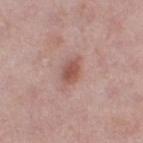notes = imaged on a skin check; not biopsied | subject = female, roughly 50 years of age | acquisition = 15 mm crop, total-body photography | anatomic site = the right thigh | image-analysis metrics = a mean CIELAB color near L≈53 a*≈23 b*≈25 and about 11 CIELAB-L* units darker than the surrounding skin; a border-irregularity index near 3/10, internal color variation of about 2.5 on a 0–10 scale, and radial color variation of about 1 | illumination = white-light.